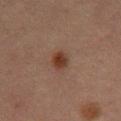Findings:
– notes · total-body-photography surveillance lesion; no biopsy
– image source · total-body-photography crop, ~15 mm field of view
– size · ≈3 mm
– automated metrics · a lesion-to-skin contrast of about 9 (normalized; higher = more distinct); a border-irregularity index near 2.5/10, a within-lesion color-variation index near 3/10, and radial color variation of about 1
– illumination · cross-polarized illumination
– location · the mid back
– subject · male, in their mid-60s